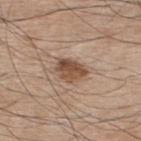lighting: white-light | automated metrics: a symmetry-axis asymmetry near 0.3; a lesion–skin lightness drop of about 13 and a normalized lesion–skin contrast near 9.5; border irregularity of about 3 on a 0–10 scale, internal color variation of about 4.5 on a 0–10 scale, and a peripheral color-asymmetry measure near 1.5 | lesion size: ≈3.5 mm | patient: male, aged 73–77 | body site: the upper back | imaging modality: ~15 mm crop, total-body skin-cancer survey.